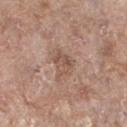This lesion was catalogued during total-body skin photography and was not selected for biopsy. A 15 mm crop from a total-body photograph taken for skin-cancer surveillance. Captured under white-light illumination. Automated tile analysis of the lesion measured an area of roughly 6 mm², a shape eccentricity near 0.6, and a shape-asymmetry score of about 0.35 (0 = symmetric). It also reported an automated nevus-likeness rating near 5 out of 100 and a detector confidence of about 100 out of 100 that the crop contains a lesion. The subject is a female in their mid-70s. The lesion is located on the left lower leg. Longest diameter approximately 3.5 mm.